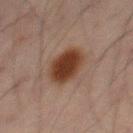This lesion was catalogued during total-body skin photography and was not selected for biopsy.
The recorded lesion diameter is about 4.5 mm.
A close-up tile cropped from a whole-body skin photograph, about 15 mm across.
The total-body-photography lesion software estimated a lesion area of about 13 mm² and a symmetry-axis asymmetry near 0.15. And it measured a lesion-to-skin contrast of about 11.5 (normalized; higher = more distinct). The software also gave a nevus-likeness score of about 100/100 and lesion-presence confidence of about 100/100.
Imaged with cross-polarized lighting.
A male patient approximately 65 years of age.
Located on the mid back.